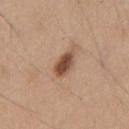Part of a total-body skin-imaging series; this lesion was reviewed on a skin check and was not flagged for biopsy. The total-body-photography lesion software estimated an area of roughly 5.5 mm², an eccentricity of roughly 0.8, and two-axis asymmetry of about 0.2. The software also gave a color-variation rating of about 3/10. And it measured a nevus-likeness score of about 100/100 and a lesion-detection confidence of about 100/100. A lesion tile, about 15 mm wide, cut from a 3D total-body photograph. On the chest. The tile uses white-light illumination. The recorded lesion diameter is about 3.5 mm. A male subject, aged around 55.